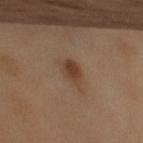{
  "biopsy_status": "not biopsied; imaged during a skin examination",
  "site": "left upper arm",
  "lighting": "cross-polarized",
  "image": {
    "source": "total-body photography crop",
    "field_of_view_mm": 15
  },
  "lesion_size": {
    "long_diameter_mm_approx": 3.5
  },
  "patient": {
    "sex": "female",
    "age_approx": 55
  }
}